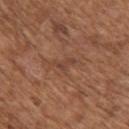Captured during whole-body skin photography for melanoma surveillance; the lesion was not biopsied.
A female patient, aged around 75.
A lesion tile, about 15 mm wide, cut from a 3D total-body photograph.
Located on the left upper arm.
About 3 mm across.